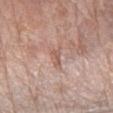* follow-up: imaged on a skin check; not biopsied
* image-analysis metrics: a shape-asymmetry score of about 0.25 (0 = symmetric); border irregularity of about 3 on a 0–10 scale, a within-lesion color-variation index near 0.5/10, and a peripheral color-asymmetry measure near 0
* body site: the right forearm
* image source: total-body-photography crop, ~15 mm field of view
* subject: female, aged approximately 65